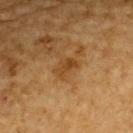patient: male, aged 83 or over
size: ~3 mm (longest diameter)
tile lighting: cross-polarized
TBP lesion metrics: a border-irregularity rating of about 3.5/10, internal color variation of about 3 on a 0–10 scale, and radial color variation of about 1; a classifier nevus-likeness of about 0/100 and a lesion-detection confidence of about 100/100
imaging modality: ~15 mm tile from a whole-body skin photo
site: the upper back